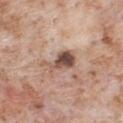The patient is a male about 65 years old. Cropped from a whole-body photographic skin survey; the tile spans about 15 mm. The lesion is located on the front of the torso. Imaged with white-light lighting.